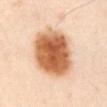Part of a total-body skin-imaging series; this lesion was reviewed on a skin check and was not flagged for biopsy. A 15 mm close-up tile from a total-body photography series done for melanoma screening. The subject is a male approximately 65 years of age. Located on the abdomen.